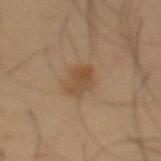The lesion was photographed on a routine skin check and not biopsied; there is no pathology result. A close-up tile cropped from a whole-body skin photograph, about 15 mm across. A male subject aged 53 to 57. Approximately 3 mm at its widest. Located on the abdomen.